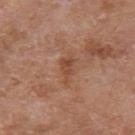| key | value |
|---|---|
| biopsy status | imaged on a skin check; not biopsied |
| illumination | white-light |
| subject | male, aged 63–67 |
| image | ~15 mm tile from a whole-body skin photo |
| site | the left upper arm |
| lesion size | about 3.5 mm |